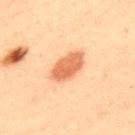Q: Was a biopsy performed?
A: total-body-photography surveillance lesion; no biopsy
Q: What is the imaging modality?
A: ~15 mm tile from a whole-body skin photo
Q: What is the anatomic site?
A: the upper back
Q: How was the tile lit?
A: cross-polarized
Q: What are the patient's age and sex?
A: male, aged around 40
Q: What is the lesion's diameter?
A: ~4.5 mm (longest diameter)